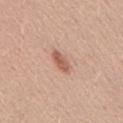Case summary:
* subject: male, aged approximately 55
* anatomic site: the back
* diameter: ≈3.5 mm
* automated lesion analysis: a lesion area of about 4.5 mm², an eccentricity of roughly 0.9, and a symmetry-axis asymmetry near 0.15; a lesion color around L≈59 a*≈23 b*≈29 in CIELAB and a normalized lesion–skin contrast near 7.5; a classifier nevus-likeness of about 80/100
* imaging modality: total-body-photography crop, ~15 mm field of view
* tile lighting: white-light